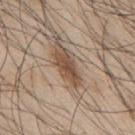Imaged during a routine full-body skin examination; the lesion was not biopsied and no histopathology is available.
This image is a 15 mm lesion crop taken from a total-body photograph.
A male patient about 45 years old.
Located on the back.
Approximately 4.5 mm at its widest.